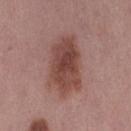Clinical impression:
The lesion was tiled from a total-body skin photograph and was not biopsied.
Context:
A female subject, aged 48 to 52. A 15 mm close-up extracted from a 3D total-body photography capture. From the right thigh. This is a white-light tile. An algorithmic analysis of the crop reported a footprint of about 22 mm², a shape eccentricity near 0.75, and a shape-asymmetry score of about 0.15 (0 = symmetric). It also reported an average lesion color of about L≈45 a*≈22 b*≈23 (CIELAB), a lesion–skin lightness drop of about 11, and a normalized border contrast of about 9. And it measured a border-irregularity rating of about 2.5/10, internal color variation of about 4.5 on a 0–10 scale, and a peripheral color-asymmetry measure near 1.5.